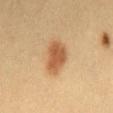<lesion>
<biopsy_status>not biopsied; imaged during a skin examination</biopsy_status>
<site>chest</site>
<patient>
  <sex>female</sex>
  <age_approx>30</age_approx>
</patient>
<lesion_size>
  <long_diameter_mm_approx>4.5</long_diameter_mm_approx>
</lesion_size>
<image>
  <source>total-body photography crop</source>
  <field_of_view_mm>15</field_of_view_mm>
</image>
<lighting>cross-polarized</lighting>
<automated_metrics>
  <nevus_likeness_0_100>100</nevus_likeness_0_100>
  <lesion_detection_confidence_0_100>100</lesion_detection_confidence_0_100>
</automated_metrics>
</lesion>